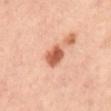- workup: total-body-photography surveillance lesion; no biopsy
- patient: female, about 35 years old
- image source: ~15 mm tile from a whole-body skin photo
- lesion size: about 3 mm
- illumination: cross-polarized
- body site: the abdomen